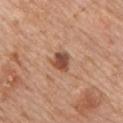Captured during whole-body skin photography for melanoma surveillance; the lesion was not biopsied. Approximately 2.5 mm at its widest. The patient is a male aged approximately 60. The lesion is located on the chest. A region of skin cropped from a whole-body photographic capture, roughly 15 mm wide.